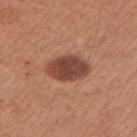Imaged during a routine full-body skin examination; the lesion was not biopsied and no histopathology is available. A 15 mm crop from a total-body photograph taken for skin-cancer surveillance. A female subject, in their mid-60s. This is a white-light tile. The lesion is located on the left upper arm. About 5 mm across.